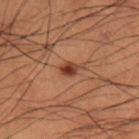follow-up=no biopsy performed (imaged during a skin exam); lighting=cross-polarized; location=the right lower leg; acquisition=~15 mm crop, total-body skin-cancer survey; patient=male, roughly 50 years of age.